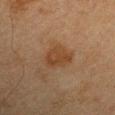Recorded during total-body skin imaging; not selected for excision or biopsy.
A region of skin cropped from a whole-body photographic capture, roughly 15 mm wide.
Located on the right upper arm.
A male subject, roughly 65 years of age.
Measured at roughly 3.5 mm in maximum diameter.
The tile uses cross-polarized illumination.